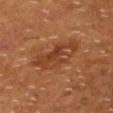{
  "biopsy_status": "not biopsied; imaged during a skin examination",
  "lesion_size": {
    "long_diameter_mm_approx": 5.5
  },
  "patient": {
    "sex": "male",
    "age_approx": 60
  },
  "image": {
    "source": "total-body photography crop",
    "field_of_view_mm": 15
  },
  "automated_metrics": {
    "cielab_L": 39,
    "cielab_a": 23,
    "cielab_b": 33,
    "vs_skin_contrast_norm": 6.5
  },
  "site": "head or neck",
  "lighting": "cross-polarized"
}